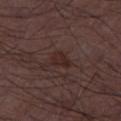workup: catalogued during a skin exam; not biopsied | body site: the right thigh | acquisition: ~15 mm tile from a whole-body skin photo | patient: male, aged around 50 | size: about 3 mm.